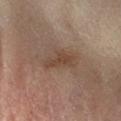No biopsy was performed on this lesion — it was imaged during a full skin examination and was not determined to be concerning.
Longest diameter approximately 4.5 mm.
A 15 mm close-up extracted from a 3D total-body photography capture.
The lesion-visualizer software estimated lesion-presence confidence of about 100/100.
Imaged with cross-polarized lighting.
A female patient approximately 65 years of age.
The lesion is on the left arm.